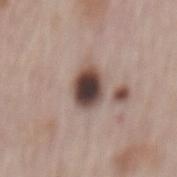{"biopsy_status": "not biopsied; imaged during a skin examination", "lesion_size": {"long_diameter_mm_approx": 4.0}, "patient": {"sex": "male", "age_approx": 65}, "automated_metrics": {"color_variation_0_10": 7.5, "peripheral_color_asymmetry": 1.5}, "image": {"source": "total-body photography crop", "field_of_view_mm": 15}, "lighting": "white-light", "site": "mid back"}